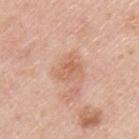No biopsy was performed on this lesion — it was imaged during a full skin examination and was not determined to be concerning.
The recorded lesion diameter is about 2.5 mm.
This is a white-light tile.
The subject is a male in their mid-40s.
Cropped from a total-body skin-imaging series; the visible field is about 15 mm.
The lesion is located on the upper back.